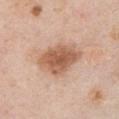The lesion was photographed on a routine skin check and not biopsied; there is no pathology result. A 15 mm close-up extracted from a 3D total-body photography capture. From the chest. A female patient aged 58 to 62.